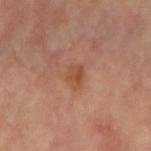{
  "lesion_size": {
    "long_diameter_mm_approx": 3.0
  },
  "image": {
    "source": "total-body photography crop",
    "field_of_view_mm": 15
  },
  "patient": {
    "sex": "female",
    "age_approx": 65
  },
  "site": "right forearm",
  "automated_metrics": {
    "area_mm2_approx": 4.5,
    "shape_asymmetry": 0.35,
    "cielab_L": 51,
    "cielab_a": 25,
    "cielab_b": 33,
    "vs_skin_darker_L": 7.0,
    "vs_skin_contrast_norm": 6.5,
    "nevus_likeness_0_100": 0,
    "lesion_detection_confidence_0_100": 100
  }
}